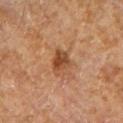Recorded during total-body skin imaging; not selected for excision or biopsy. Located on the left forearm. A 15 mm crop from a total-body photograph taken for skin-cancer surveillance. A male patient aged around 65.